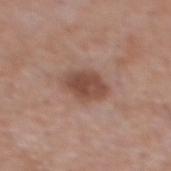The lesion was photographed on a routine skin check and not biopsied; there is no pathology result.
The recorded lesion diameter is about 4 mm.
The lesion is on the back.
Cropped from a whole-body photographic skin survey; the tile spans about 15 mm.
A male patient aged around 55.